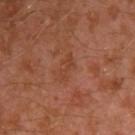Context:
The lesion-visualizer software estimated a mean CIELAB color near L≈40 a*≈24 b*≈31, roughly 5 lightness units darker than nearby skin, and a normalized border contrast of about 5. And it measured an automated nevus-likeness rating near 0 out of 100 and a detector confidence of about 100 out of 100 that the crop contains a lesion. This image is a 15 mm lesion crop taken from a total-body photograph. This is a cross-polarized tile. The lesion is on the left arm. A male subject approximately 30 years of age.